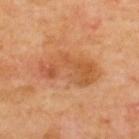  biopsy_status: not biopsied; imaged during a skin examination
  automated_metrics:
    area_mm2_approx: 16.0
    eccentricity: 0.9
    shape_asymmetry: 0.35
  site: upper back
  patient:
    sex: male
    age_approx: 70
  image:
    source: total-body photography crop
    field_of_view_mm: 15
  lighting: cross-polarized